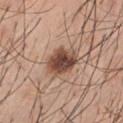The lesion was photographed on a routine skin check and not biopsied; there is no pathology result. Imaged with white-light lighting. A roughly 15 mm field-of-view crop from a total-body skin photograph. The subject is a male about 45 years old. On the front of the torso. The total-body-photography lesion software estimated a lesion area of about 11 mm², an eccentricity of roughly 0.65, and a shape-asymmetry score of about 0.15 (0 = symmetric).